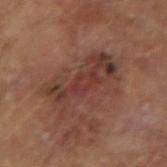Case summary:
– subject — male, roughly 65 years of age
– tile lighting — cross-polarized illumination
– size — about 7.5 mm
– site — the right upper arm
– image — 15 mm crop, total-body photography